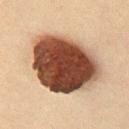Clinical impression: Recorded during total-body skin imaging; not selected for excision or biopsy. Image and clinical context: Captured under cross-polarized illumination. A 15 mm crop from a total-body photograph taken for skin-cancer surveillance. A male subject aged approximately 60. Approximately 8.5 mm at its widest. Automated tile analysis of the lesion measured a footprint of about 45 mm², an outline eccentricity of about 0.6 (0 = round, 1 = elongated), and a shape-asymmetry score of about 0.15 (0 = symmetric). The analysis additionally found an average lesion color of about L≈37 a*≈19 b*≈26 (CIELAB), roughly 24 lightness units darker than nearby skin, and a normalized border contrast of about 17.5. And it measured a nevus-likeness score of about 100/100 and lesion-presence confidence of about 100/100. On the front of the torso.